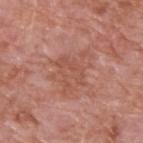Measured at roughly 5 mm in maximum diameter.
From the upper back.
The lesion-visualizer software estimated a lesion area of about 14 mm², an eccentricity of roughly 0.6, and a symmetry-axis asymmetry near 0.5. The software also gave a classifier nevus-likeness of about 0/100.
A close-up tile cropped from a whole-body skin photograph, about 15 mm across.
A male subject, roughly 60 years of age.
Imaged with white-light lighting.